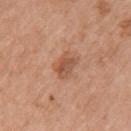The lesion was tiled from a total-body skin photograph and was not biopsied. Automated tile analysis of the lesion measured an area of roughly 6.5 mm². The lesion is on the left upper arm. A 15 mm close-up tile from a total-body photography series done for melanoma screening. The subject is a female aged around 70.